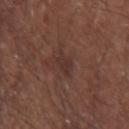A 15 mm crop from a total-body photograph taken for skin-cancer surveillance. The patient is a male aged around 65. The lesion's longest dimension is about 2.5 mm. The lesion is located on the right upper arm.